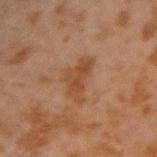Clinical impression:
The lesion was photographed on a routine skin check and not biopsied; there is no pathology result.
Image and clinical context:
The lesion is located on the arm. The tile uses cross-polarized illumination. Measured at roughly 5 mm in maximum diameter. A 15 mm close-up extracted from a 3D total-body photography capture. A male subject aged approximately 45.